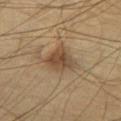Part of a total-body skin-imaging series; this lesion was reviewed on a skin check and was not flagged for biopsy. A lesion tile, about 15 mm wide, cut from a 3D total-body photograph. On the chest. Measured at roughly 3.5 mm in maximum diameter. A male subject, roughly 70 years of age. The lesion-visualizer software estimated roughly 10 lightness units darker than nearby skin and a normalized lesion–skin contrast near 8. And it measured a color-variation rating of about 5/10 and a peripheral color-asymmetry measure near 2. This is a cross-polarized tile.